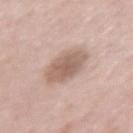Q: Is there a histopathology result?
A: imaged on a skin check; not biopsied